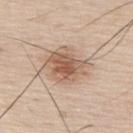  biopsy_status: not biopsied; imaged during a skin examination
  automated_metrics:
    area_mm2_approx: 16.0
    eccentricity: 0.6
    shape_asymmetry: 0.2
    cielab_L: 59
    cielab_a: 17
    cielab_b: 29
    vs_skin_darker_L: 12.0
    vs_skin_contrast_norm: 8.0
    nevus_likeness_0_100: 85
    lesion_detection_confidence_0_100: 100
  lesion_size:
    long_diameter_mm_approx: 5.5
  patient:
    sex: male
    age_approx: 75
  image:
    source: total-body photography crop
    field_of_view_mm: 15
  lighting: white-light
  site: upper back